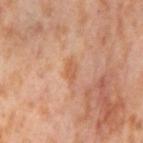This lesion was catalogued during total-body skin photography and was not selected for biopsy. A female patient, about 55 years old. From the left thigh. About 2.5 mm across. Cropped from a total-body skin-imaging series; the visible field is about 15 mm. Captured under cross-polarized illumination.